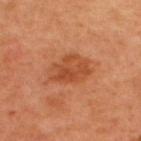The tile uses cross-polarized illumination. Measured at roughly 5 mm in maximum diameter. The subject is a male roughly 50 years of age. The lesion is on the upper back. An algorithmic analysis of the crop reported a border-irregularity index near 2/10. A close-up tile cropped from a whole-body skin photograph, about 15 mm across.